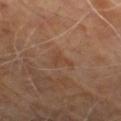{"lesion_size": {"long_diameter_mm_approx": 2.5}, "lighting": "cross-polarized", "site": "right thigh", "patient": {"age_approx": 65}, "image": {"source": "total-body photography crop", "field_of_view_mm": 15}}